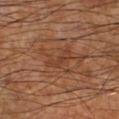biopsy_status: not biopsied; imaged during a skin examination